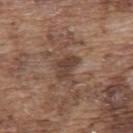automated_metrics:
  eccentricity: 0.8
  shape_asymmetry: 0.45
  lesion_detection_confidence_0_100: 100
site: upper back
lighting: white-light
patient:
  sex: male
  age_approx: 75
image:
  source: total-body photography crop
  field_of_view_mm: 15
lesion_size:
  long_diameter_mm_approx: 3.0